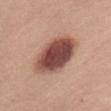No biopsy was performed on this lesion — it was imaged during a full skin examination and was not determined to be concerning. A female subject aged 48 to 52. From the left upper arm. Cropped from a total-body skin-imaging series; the visible field is about 15 mm. Captured under white-light illumination. About 6.5 mm across.